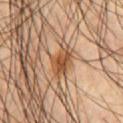Notes:
* workup: total-body-photography surveillance lesion; no biopsy
* imaging modality: ~15 mm tile from a whole-body skin photo
* patient: male, aged 58–62
* location: the chest
* automated metrics: a within-lesion color-variation index near 4.5/10 and peripheral color asymmetry of about 1.5
* lighting: cross-polarized illumination
* size: about 4 mm